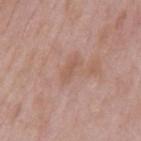{
  "site": "mid back",
  "patient": {
    "sex": "male",
    "age_approx": 55
  },
  "lighting": "white-light",
  "image": {
    "source": "total-body photography crop",
    "field_of_view_mm": 15
  }
}